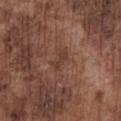{"lesion_size": {"long_diameter_mm_approx": 3.0}, "image": {"source": "total-body photography crop", "field_of_view_mm": 15}, "lighting": "white-light", "site": "front of the torso", "patient": {"sex": "male", "age_approx": 75}}